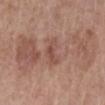Captured during whole-body skin photography for melanoma surveillance; the lesion was not biopsied. A female patient, aged approximately 65. A lesion tile, about 15 mm wide, cut from a 3D total-body photograph. Longest diameter approximately 3 mm. The total-body-photography lesion software estimated a footprint of about 4.5 mm², an eccentricity of roughly 0.65, and two-axis asymmetry of about 0.5. The analysis additionally found a lesion color around L≈49 a*≈22 b*≈26 in CIELAB, about 8 CIELAB-L* units darker than the surrounding skin, and a normalized lesion–skin contrast near 6. It also reported a color-variation rating of about 3/10 and peripheral color asymmetry of about 1. The software also gave a nevus-likeness score of about 0/100 and a lesion-detection confidence of about 100/100. From the left lower leg. Imaged with white-light lighting.